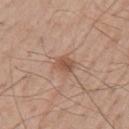Findings:
– biopsy status — no biopsy performed (imaged during a skin exam)